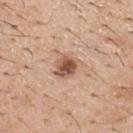Recorded during total-body skin imaging; not selected for excision or biopsy.
A male patient, in their 40s.
Approximately 3 mm at its widest.
Cropped from a whole-body photographic skin survey; the tile spans about 15 mm.
Located on the upper back.
This is a white-light tile.